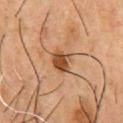biopsy_status: not biopsied; imaged during a skin examination
lighting: cross-polarized
lesion_size:
  long_diameter_mm_approx: 3.0
image:
  source: total-body photography crop
  field_of_view_mm: 15
automated_metrics:
  area_mm2_approx: 6.0
  eccentricity: 0.5
  shape_asymmetry: 0.25
  vs_skin_darker_L: 14.0
  vs_skin_contrast_norm: 9.5
  nevus_likeness_0_100: 95
  lesion_detection_confidence_0_100: 100
patient:
  sex: male
  age_approx: 55
site: chest